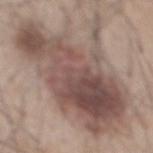Assessment:
Imaged during a routine full-body skin examination; the lesion was not biopsied and no histopathology is available.
Clinical summary:
An algorithmic analysis of the crop reported an area of roughly 75 mm², a shape eccentricity near 0.85, and two-axis asymmetry of about 0.3. It also reported a classifier nevus-likeness of about 75/100 and lesion-presence confidence of about 100/100. The lesion is located on the mid back. About 14 mm across. The tile uses white-light illumination. A lesion tile, about 15 mm wide, cut from a 3D total-body photograph. The patient is a male aged 43–47.